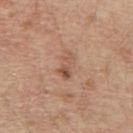Impression: Recorded during total-body skin imaging; not selected for excision or biopsy. Context: This is a white-light tile. Automated tile analysis of the lesion measured a border-irregularity rating of about 5.5/10 and peripheral color asymmetry of about 2. Measured at roughly 3.5 mm in maximum diameter. A male subject, in their 70s. The lesion is located on the back. Cropped from a total-body skin-imaging series; the visible field is about 15 mm.